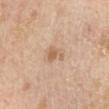biopsy status: total-body-photography surveillance lesion; no biopsy | lesion size: ≈2.5 mm | site: the left lower leg | acquisition: 15 mm crop, total-body photography | TBP lesion metrics: a lesion area of about 3.5 mm², an outline eccentricity of about 0.7 (0 = round, 1 = elongated), and two-axis asymmetry of about 0.45; a mean CIELAB color near L≈59 a*≈17 b*≈33; a border-irregularity rating of about 5/10, a within-lesion color-variation index near 1.5/10, and peripheral color asymmetry of about 0.5; an automated nevus-likeness rating near 0 out of 100 and lesion-presence confidence of about 100/100 | patient: male, aged 48 to 52.